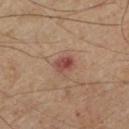biopsy_status: not biopsied; imaged during a skin examination
image:
  source: total-body photography crop
  field_of_view_mm: 15
patient:
  sex: male
  age_approx: 70
site: left lower leg